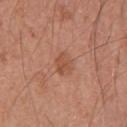Findings:
– notes · total-body-photography surveillance lesion; no biopsy
– lesion size · about 2.5 mm
– automated metrics · a footprint of about 4 mm² and a shape eccentricity near 0.65; an average lesion color of about L≈51 a*≈25 b*≈32 (CIELAB), a lesion–skin lightness drop of about 8, and a lesion-to-skin contrast of about 6 (normalized; higher = more distinct); a classifier nevus-likeness of about 5/100 and a lesion-detection confidence of about 100/100
– acquisition · ~15 mm tile from a whole-body skin photo
– subject · male, aged approximately 45
– site · the upper back
– tile lighting · white-light illumination